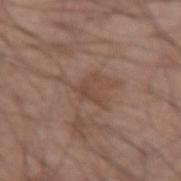No biopsy was performed on this lesion — it was imaged during a full skin examination and was not determined to be concerning. Located on the left forearm. A male patient, in their mid- to late 60s. A roughly 15 mm field-of-view crop from a total-body skin photograph.